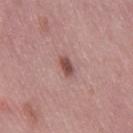The lesion was photographed on a routine skin check and not biopsied; there is no pathology result. A lesion tile, about 15 mm wide, cut from a 3D total-body photograph. Imaged with white-light lighting. A female subject aged 48 to 52. The recorded lesion diameter is about 2.5 mm. The lesion is located on the right thigh.